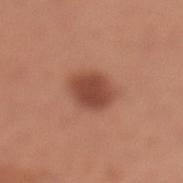Assessment: No biopsy was performed on this lesion — it was imaged during a full skin examination and was not determined to be concerning. Clinical summary: The lesion is located on the left lower leg. Automated image analysis of the tile measured a mean CIELAB color near L≈46 a*≈25 b*≈30 and roughly 12 lightness units darker than nearby skin. And it measured border irregularity of about 1.5 on a 0–10 scale and peripheral color asymmetry of about 0.5. And it measured lesion-presence confidence of about 100/100. Imaged with white-light lighting. A female subject, aged 28 to 32. A roughly 15 mm field-of-view crop from a total-body skin photograph. About 4 mm across.